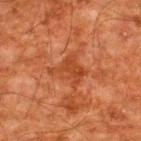<lesion>
<biopsy_status>not biopsied; imaged during a skin examination</biopsy_status>
<image>
  <source>total-body photography crop</source>
  <field_of_view_mm>15</field_of_view_mm>
</image>
<patient>
  <sex>male</sex>
  <age_approx>60</age_approx>
</patient>
<lighting>cross-polarized</lighting>
<automated_metrics>
  <area_mm2_approx>7.5</area_mm2_approx>
  <eccentricity>0.6</eccentricity>
  <shape_asymmetry>0.45</shape_asymmetry>
  <cielab_L>35</cielab_L>
  <cielab_a>25</cielab_a>
  <cielab_b>33</cielab_b>
  <vs_skin_contrast_norm>6.0</vs_skin_contrast_norm>
  <nevus_likeness_0_100>0</nevus_likeness_0_100>
  <lesion_detection_confidence_0_100>100</lesion_detection_confidence_0_100>
</automated_metrics>
<site>back</site>
</lesion>